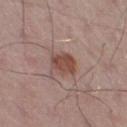patient: male, about 55 years old; size: about 3 mm; imaging modality: ~15 mm crop, total-body skin-cancer survey; anatomic site: the right thigh.